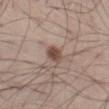workup=no biopsy performed (imaged during a skin exam); subject=male, about 55 years old; imaging modality=total-body-photography crop, ~15 mm field of view; body site=the left thigh.